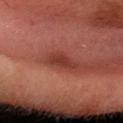Imaged during a routine full-body skin examination; the lesion was not biopsied and no histopathology is available. Cropped from a total-body skin-imaging series; the visible field is about 15 mm. A male subject aged 53 to 57. Automated image analysis of the tile measured a footprint of about 4 mm², an eccentricity of roughly 0.75, and a shape-asymmetry score of about 0.25 (0 = symmetric). The software also gave an average lesion color of about L≈29 a*≈24 b*≈23 (CIELAB) and about 8 CIELAB-L* units darker than the surrounding skin. It also reported a border-irregularity index near 2/10, a within-lesion color-variation index near 1/10, and a peripheral color-asymmetry measure near 0.5. On the head or neck.